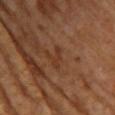No biopsy was performed on this lesion — it was imaged during a full skin examination and was not determined to be concerning.
Automated image analysis of the tile measured an average lesion color of about L≈34 a*≈21 b*≈30 (CIELAB), roughly 5 lightness units darker than nearby skin, and a normalized lesion–skin contrast near 5. And it measured a border-irregularity index near 6/10, internal color variation of about 0 on a 0–10 scale, and a peripheral color-asymmetry measure near 0. It also reported a nevus-likeness score of about 0/100 and a lesion-detection confidence of about 100/100.
The patient is a female roughly 65 years of age.
The lesion is located on the upper back.
A roughly 15 mm field-of-view crop from a total-body skin photograph.
The lesion's longest dimension is about 2.5 mm.